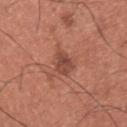Imaged during a routine full-body skin examination; the lesion was not biopsied and no histopathology is available.
An algorithmic analysis of the crop reported a classifier nevus-likeness of about 65/100 and a detector confidence of about 100 out of 100 that the crop contains a lesion.
A region of skin cropped from a whole-body photographic capture, roughly 15 mm wide.
This is a white-light tile.
The lesion is on the back.
The patient is a male about 35 years old.
The recorded lesion diameter is about 2.5 mm.